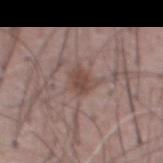This lesion was catalogued during total-body skin photography and was not selected for biopsy.
A male patient aged 53–57.
Longest diameter approximately 3 mm.
Automated tile analysis of the lesion measured a classifier nevus-likeness of about 85/100.
The lesion is located on the abdomen.
Captured under white-light illumination.
A 15 mm crop from a total-body photograph taken for skin-cancer surveillance.